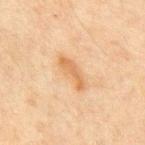Clinical impression: Part of a total-body skin-imaging series; this lesion was reviewed on a skin check and was not flagged for biopsy. Clinical summary: On the abdomen. Measured at roughly 4.5 mm in maximum diameter. A male patient, aged around 65. A region of skin cropped from a whole-body photographic capture, roughly 15 mm wide. An algorithmic analysis of the crop reported an area of roughly 5 mm² and two-axis asymmetry of about 0.45. The software also gave a mean CIELAB color near L≈57 a*≈19 b*≈37, about 9 CIELAB-L* units darker than the surrounding skin, and a lesion-to-skin contrast of about 7 (normalized; higher = more distinct). The software also gave an automated nevus-likeness rating near 5 out of 100 and a detector confidence of about 100 out of 100 that the crop contains a lesion.